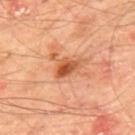Impression: The lesion was tiled from a total-body skin photograph and was not biopsied. Context: The recorded lesion diameter is about 3.5 mm. Located on the mid back. A male patient aged approximately 65. A close-up tile cropped from a whole-body skin photograph, about 15 mm across.